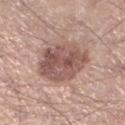Assessment: Imaged during a routine full-body skin examination; the lesion was not biopsied and no histopathology is available. Context: From the left lower leg. Imaged with white-light lighting. A close-up tile cropped from a whole-body skin photograph, about 15 mm across. Approximately 6 mm at its widest. Automated tile analysis of the lesion measured a mean CIELAB color near L≈53 a*≈20 b*≈23, about 13 CIELAB-L* units darker than the surrounding skin, and a normalized border contrast of about 8.5. The analysis additionally found border irregularity of about 2 on a 0–10 scale, internal color variation of about 6 on a 0–10 scale, and peripheral color asymmetry of about 2.5. And it measured lesion-presence confidence of about 100/100. A male patient roughly 40 years of age.